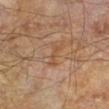Imaged during a routine full-body skin examination; the lesion was not biopsied and no histopathology is available.
The subject is a male aged 63–67.
A region of skin cropped from a whole-body photographic capture, roughly 15 mm wide.